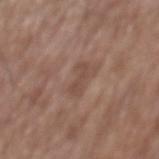Captured during whole-body skin photography for melanoma surveillance; the lesion was not biopsied. A close-up tile cropped from a whole-body skin photograph, about 15 mm across. Captured under white-light illumination. The lesion is located on the back. A male subject about 75 years old. About 3 mm across. An algorithmic analysis of the crop reported border irregularity of about 6.5 on a 0–10 scale, a color-variation rating of about 0/10, and a peripheral color-asymmetry measure near 0. It also reported an automated nevus-likeness rating near 0 out of 100 and a detector confidence of about 100 out of 100 that the crop contains a lesion.